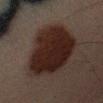notes: catalogued during a skin exam; not biopsied
automated metrics: a footprint of about 36 mm² and a shape eccentricity near 0.65; an average lesion color of about L≈16 a*≈13 b*≈15 (CIELAB) and about 11 CIELAB-L* units darker than the surrounding skin; an automated nevus-likeness rating near 95 out of 100 and a lesion-detection confidence of about 100/100
patient: male, in their 50s
lighting: cross-polarized illumination
diameter: ≈8 mm
imaging modality: ~15 mm crop, total-body skin-cancer survey
anatomic site: the left upper arm